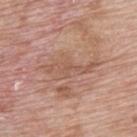Clinical impression:
Imaged during a routine full-body skin examination; the lesion was not biopsied and no histopathology is available.
Background:
A male subject in their 70s. Located on the upper back. A region of skin cropped from a whole-body photographic capture, roughly 15 mm wide.